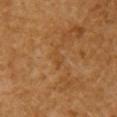Background: A region of skin cropped from a whole-body photographic capture, roughly 15 mm wide. The subject is a female approximately 55 years of age. On the right upper arm.